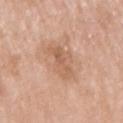Case summary:
• notes — imaged on a skin check; not biopsied
• TBP lesion metrics — an eccentricity of roughly 0.8 and two-axis asymmetry of about 0.35; an average lesion color of about L≈60 a*≈20 b*≈32 (CIELAB), roughly 8 lightness units darker than nearby skin, and a lesion-to-skin contrast of about 5.5 (normalized; higher = more distinct)
• body site — the right upper arm
• patient — female, in their mid- to late 60s
• imaging modality — ~15 mm crop, total-body skin-cancer survey
• lesion size — ≈4.5 mm